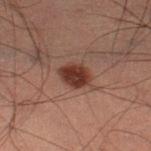Impression:
The lesion was tiled from a total-body skin photograph and was not biopsied.
Image and clinical context:
This is a cross-polarized tile. Measured at roughly 3.5 mm in maximum diameter. On the left lower leg. A male subject, aged approximately 60. A close-up tile cropped from a whole-body skin photograph, about 15 mm across. An algorithmic analysis of the crop reported a shape eccentricity near 0.65 and a symmetry-axis asymmetry near 0.15. The software also gave a lesion color around L≈26 a*≈18 b*≈20 in CIELAB, about 11 CIELAB-L* units darker than the surrounding skin, and a lesion-to-skin contrast of about 11 (normalized; higher = more distinct). The analysis additionally found a detector confidence of about 100 out of 100 that the crop contains a lesion.